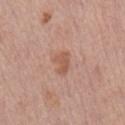follow-up = total-body-photography surveillance lesion; no biopsy | acquisition = total-body-photography crop, ~15 mm field of view | lesion size = ≈2.5 mm | subject = male, in their mid-70s | automated metrics = a border-irregularity rating of about 4/10, a color-variation rating of about 0.5/10, and radial color variation of about 0 | tile lighting = white-light illumination | body site = the mid back.